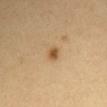The lesion was tiled from a total-body skin photograph and was not biopsied.
Cropped from a total-body skin-imaging series; the visible field is about 15 mm.
A female patient, in their mid-50s.
From the chest.
Captured under cross-polarized illumination.
The recorded lesion diameter is about 2 mm.